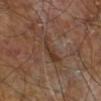The lesion was photographed on a routine skin check and not biopsied; there is no pathology result.